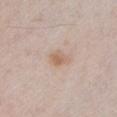Context: The subject is a male roughly 25 years of age. Measured at roughly 2.5 mm in maximum diameter. A 15 mm close-up tile from a total-body photography series done for melanoma screening. The tile uses white-light illumination. Located on the chest.